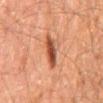Q: Was a biopsy performed?
A: catalogued during a skin exam; not biopsied
Q: What is the anatomic site?
A: the mid back
Q: What kind of image is this?
A: 15 mm crop, total-body photography
Q: Patient demographics?
A: male, aged 58 to 62